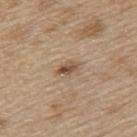biopsy_status: not biopsied; imaged during a skin examination
image:
  source: total-body photography crop
  field_of_view_mm: 15
patient:
  sex: male
  age_approx: 70
lesion_size:
  long_diameter_mm_approx: 2.5
lighting: white-light
automated_metrics:
  nevus_likeness_0_100: 85
  lesion_detection_confidence_0_100: 100
site: mid back